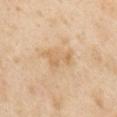biopsy status: imaged on a skin check; not biopsied
body site: the right upper arm
image source: 15 mm crop, total-body photography
illumination: cross-polarized
subject: male, aged 53 to 57
diameter: ~5 mm (longest diameter)